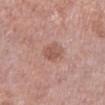Impression:
Imaged during a routine full-body skin examination; the lesion was not biopsied and no histopathology is available.
Acquisition and patient details:
Automated image analysis of the tile measured an outline eccentricity of about 0.6 (0 = round, 1 = elongated) and a shape-asymmetry score of about 0.25 (0 = symmetric). And it measured a border-irregularity rating of about 2.5/10 and a peripheral color-asymmetry measure near 1. Imaged with white-light lighting. The subject is a male in their mid- to late 70s. The lesion's longest dimension is about 3 mm. A close-up tile cropped from a whole-body skin photograph, about 15 mm across. The lesion is located on the left lower leg.